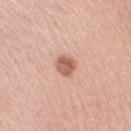{
  "lighting": "white-light",
  "image": {
    "source": "total-body photography crop",
    "field_of_view_mm": 15
  },
  "site": "right upper arm",
  "patient": {
    "sex": "female",
    "age_approx": 60
  },
  "lesion_size": {
    "long_diameter_mm_approx": 2.5
  }
}